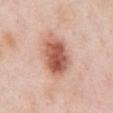Captured during whole-body skin photography for melanoma surveillance; the lesion was not biopsied.
A male subject aged 53 to 57.
A close-up tile cropped from a whole-body skin photograph, about 15 mm across.
About 6 mm across.
From the abdomen.